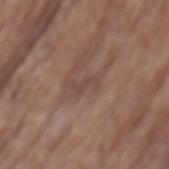Q: Was this lesion biopsied?
A: catalogued during a skin exam; not biopsied